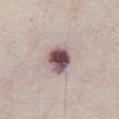Recorded during total-body skin imaging; not selected for excision or biopsy.
Imaged with white-light lighting.
A male patient aged 78–82.
About 3.5 mm across.
Cropped from a total-body skin-imaging series; the visible field is about 15 mm.
Located on the abdomen.